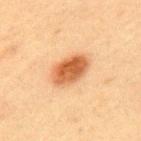Recorded during total-body skin imaging; not selected for excision or biopsy. A lesion tile, about 15 mm wide, cut from a 3D total-body photograph. A male subject approximately 60 years of age. The lesion is located on the upper back.